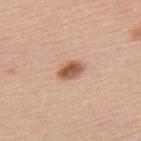Assessment: The lesion was tiled from a total-body skin photograph and was not biopsied. Image and clinical context: From the upper back. The patient is a female approximately 65 years of age. This is a white-light tile. A 15 mm close-up tile from a total-body photography series done for melanoma screening. Approximately 3 mm at its widest.